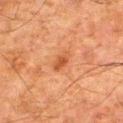notes: no biopsy performed (imaged during a skin exam) | image source: total-body-photography crop, ~15 mm field of view | TBP lesion metrics: a lesion area of about 3.5 mm², a shape eccentricity near 0.8, and a symmetry-axis asymmetry near 0.2; a lesion color around L≈43 a*≈24 b*≈34 in CIELAB, roughly 8 lightness units darker than nearby skin, and a normalized lesion–skin contrast near 6.5; a classifier nevus-likeness of about 30/100 | body site: the right thigh | tile lighting: cross-polarized illumination | subject: male, about 80 years old | lesion size: ~3 mm (longest diameter).